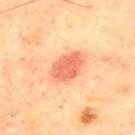follow-up=imaged on a skin check; not biopsied | lighting=cross-polarized illumination | patient=male, in their mid-60s | diameter=~4.5 mm (longest diameter) | body site=the chest | image source=~15 mm tile from a whole-body skin photo.